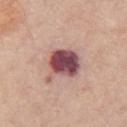Clinical impression: Imaged during a routine full-body skin examination; the lesion was not biopsied and no histopathology is available. Clinical summary: The lesion-visualizer software estimated a lesion area of about 12 mm² and an outline eccentricity of about 0.5 (0 = round, 1 = elongated). The analysis additionally found an automated nevus-likeness rating near 30 out of 100 and a detector confidence of about 100 out of 100 that the crop contains a lesion. Imaged with white-light lighting. This image is a 15 mm lesion crop taken from a total-body photograph. Approximately 4.5 mm at its widest. A female patient, aged around 70. The lesion is located on the chest.